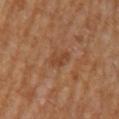The lesion was tiled from a total-body skin photograph and was not biopsied. On the left upper arm. A male subject in their mid- to late 60s. The recorded lesion diameter is about 3 mm. The tile uses cross-polarized illumination. A roughly 15 mm field-of-view crop from a total-body skin photograph.